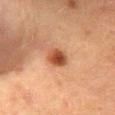Assessment: Imaged during a routine full-body skin examination; the lesion was not biopsied and no histopathology is available. Background: A female subject about 50 years old. On the abdomen. Cropped from a total-body skin-imaging series; the visible field is about 15 mm.